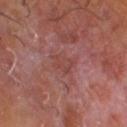Captured during whole-body skin photography for melanoma surveillance; the lesion was not biopsied.
Captured under cross-polarized illumination.
The patient is a male aged around 60.
A 15 mm close-up tile from a total-body photography series done for melanoma screening.
Measured at roughly 3 mm in maximum diameter.
An algorithmic analysis of the crop reported a footprint of about 5 mm², a shape eccentricity near 0.6, and a symmetry-axis asymmetry near 0.35. The analysis additionally found a lesion–skin lightness drop of about 5 and a normalized lesion–skin contrast near 4.5. And it measured a border-irregularity rating of about 4/10, a color-variation rating of about 2/10, and a peripheral color-asymmetry measure near 1. The analysis additionally found a nevus-likeness score of about 0/100 and lesion-presence confidence of about 90/100.
From the leg.